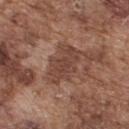Part of a total-body skin-imaging series; this lesion was reviewed on a skin check and was not flagged for biopsy. A male patient roughly 75 years of age. The tile uses white-light illumination. A roughly 15 mm field-of-view crop from a total-body skin photograph. The total-body-photography lesion software estimated two-axis asymmetry of about 0.35. The lesion is located on the upper back.